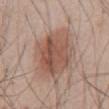Notes:
– follow-up — imaged on a skin check; not biopsied
– location — the abdomen
– size — ~6.5 mm (longest diameter)
– image-analysis metrics — an area of roughly 29 mm², a shape eccentricity near 0.5, and a symmetry-axis asymmetry near 0.15; a mean CIELAB color near L≈53 a*≈19 b*≈26 and a normalized lesion–skin contrast near 7.5
– image — ~15 mm crop, total-body skin-cancer survey
– subject — male, roughly 45 years of age